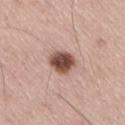Part of a total-body skin-imaging series; this lesion was reviewed on a skin check and was not flagged for biopsy. A region of skin cropped from a whole-body photographic capture, roughly 15 mm wide. The patient is a male aged 53–57. The lesion's longest dimension is about 3 mm. The total-body-photography lesion software estimated an average lesion color of about L≈49 a*≈21 b*≈25 (CIELAB) and a normalized border contrast of about 12.5. It also reported border irregularity of about 1.5 on a 0–10 scale, internal color variation of about 4.5 on a 0–10 scale, and peripheral color asymmetry of about 1. It also reported an automated nevus-likeness rating near 90 out of 100. This is a white-light tile. From the right thigh.